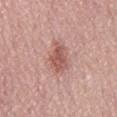Captured during whole-body skin photography for melanoma surveillance; the lesion was not biopsied.